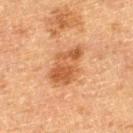patient: male, about 75 years old | automated lesion analysis: a lesion area of about 13 mm² and an eccentricity of roughly 0.8; about 9 CIELAB-L* units darker than the surrounding skin and a normalized border contrast of about 7.5 | illumination: cross-polarized illumination | lesion diameter: ~5.5 mm (longest diameter) | image: 15 mm crop, total-body photography | anatomic site: the left thigh.